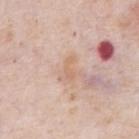Clinical impression:
Imaged during a routine full-body skin examination; the lesion was not biopsied and no histopathology is available.